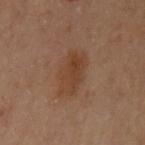Clinical summary: Longest diameter approximately 4.5 mm. A female subject in their 60s. A close-up tile cropped from a whole-body skin photograph, about 15 mm across. This is a cross-polarized tile. On the right arm.